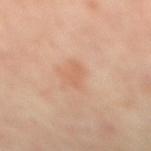Part of a total-body skin-imaging series; this lesion was reviewed on a skin check and was not flagged for biopsy. Cropped from a whole-body photographic skin survey; the tile spans about 15 mm. From the right lower leg. A female patient, aged around 50. The lesion's longest dimension is about 3 mm. Imaged with cross-polarized lighting.